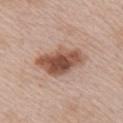biopsy_status: not biopsied; imaged during a skin examination
patient:
  sex: male
  age_approx: 65
image:
  source: total-body photography crop
  field_of_view_mm: 15
site: chest